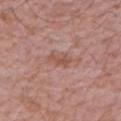<case>
<biopsy_status>not biopsied; imaged during a skin examination</biopsy_status>
<lesion_size>
  <long_diameter_mm_approx>2.5</long_diameter_mm_approx>
</lesion_size>
<site>left upper arm</site>
<image>
  <source>total-body photography crop</source>
  <field_of_view_mm>15</field_of_view_mm>
</image>
<automated_metrics>
  <cielab_L>52</cielab_L>
  <cielab_a>23</cielab_a>
  <cielab_b>26</cielab_b>
  <vs_skin_darker_L>8.0</vs_skin_darker_L>
  <vs_skin_contrast_norm>6.5</vs_skin_contrast_norm>
  <border_irregularity_0_10>4.0</border_irregularity_0_10>
  <color_variation_0_10>0.5</color_variation_0_10>
  <peripheral_color_asymmetry>0.0</peripheral_color_asymmetry>
  <nevus_likeness_0_100>0</nevus_likeness_0_100>
  <lesion_detection_confidence_0_100>100</lesion_detection_confidence_0_100>
</automated_metrics>
<patient>
  <sex>male</sex>
  <age_approx>65</age_approx>
</patient>
<lighting>white-light</lighting>
</case>